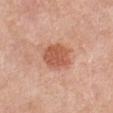Part of a total-body skin-imaging series; this lesion was reviewed on a skin check and was not flagged for biopsy. The subject is a female aged 53–57. Cropped from a total-body skin-imaging series; the visible field is about 15 mm. The tile uses white-light illumination. Automated tile analysis of the lesion measured a within-lesion color-variation index near 3/10 and peripheral color asymmetry of about 1. The lesion is on the chest.